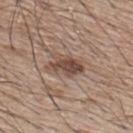Imaged during a routine full-body skin examination; the lesion was not biopsied and no histopathology is available.
A 15 mm close-up tile from a total-body photography series done for melanoma screening.
The lesion is located on the upper back.
A male subject roughly 65 years of age.